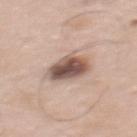<tbp_lesion>
  <biopsy_status>not biopsied; imaged during a skin examination</biopsy_status>
  <lighting>white-light</lighting>
  <image>
    <source>total-body photography crop</source>
    <field_of_view_mm>15</field_of_view_mm>
  </image>
  <lesion_size>
    <long_diameter_mm_approx>4.0</long_diameter_mm_approx>
  </lesion_size>
  <patient>
    <sex>male</sex>
    <age_approx>60</age_approx>
  </patient>
  <automated_metrics>
    <vs_skin_darker_L>18.0</vs_skin_darker_L>
    <vs_skin_contrast_norm>11.5</vs_skin_contrast_norm>
    <border_irregularity_0_10>2.0</border_irregularity_0_10>
    <peripheral_color_asymmetry>1.5</peripheral_color_asymmetry>
  </automated_metrics>
  <site>upper back</site>
</tbp_lesion>